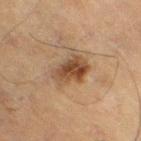This lesion was catalogued during total-body skin photography and was not selected for biopsy. On the right thigh. Imaged with cross-polarized lighting. The subject is a male roughly 70 years of age. Automated tile analysis of the lesion measured an area of roughly 10 mm² and a shape eccentricity near 0.7. And it measured border irregularity of about 2.5 on a 0–10 scale and radial color variation of about 2. This image is a 15 mm lesion crop taken from a total-body photograph.